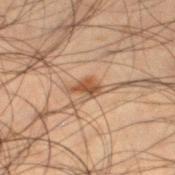Q: Was this lesion biopsied?
A: catalogued during a skin exam; not biopsied
Q: Illumination type?
A: cross-polarized illumination
Q: Automated lesion metrics?
A: an average lesion color of about L≈38 a*≈17 b*≈28 (CIELAB), a lesion–skin lightness drop of about 10, and a normalized lesion–skin contrast near 9
Q: What are the patient's age and sex?
A: male, aged approximately 50
Q: Lesion size?
A: ≈2.5 mm
Q: What is the imaging modality?
A: 15 mm crop, total-body photography
Q: Where on the body is the lesion?
A: the left lower leg